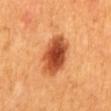<case>
<biopsy_status>not biopsied; imaged during a skin examination</biopsy_status>
<patient>
  <sex>male</sex>
</patient>
<image>
  <source>total-body photography crop</source>
  <field_of_view_mm>15</field_of_view_mm>
</image>
<lesion_size>
  <long_diameter_mm_approx>5.5</long_diameter_mm_approx>
</lesion_size>
<site>mid back</site>
<lighting>cross-polarized</lighting>
</case>